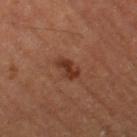{"biopsy_status": "not biopsied; imaged during a skin examination", "patient": {"sex": "male", "age_approx": 55}, "site": "left thigh", "image": {"source": "total-body photography crop", "field_of_view_mm": 15}, "lesion_size": {"long_diameter_mm_approx": 3.5}, "automated_metrics": {"cielab_L": 32, "cielab_a": 23, "cielab_b": 29, "vs_skin_darker_L": 9.0}, "lighting": "cross-polarized"}